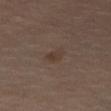Notes:
- biopsy status · total-body-photography surveillance lesion; no biopsy
- illumination · cross-polarized
- TBP lesion metrics · a lesion color around L≈36 a*≈13 b*≈23 in CIELAB, a lesion–skin lightness drop of about 5, and a normalized lesion–skin contrast near 5.5; a peripheral color-asymmetry measure near 1; a nevus-likeness score of about 10/100 and lesion-presence confidence of about 100/100
- patient · male, roughly 65 years of age
- lesion size · ~2.5 mm (longest diameter)
- anatomic site · the right thigh
- image · total-body-photography crop, ~15 mm field of view